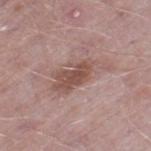<tbp_lesion>
<biopsy_status>not biopsied; imaged during a skin examination</biopsy_status>
<site>left thigh</site>
<image>
  <source>total-body photography crop</source>
  <field_of_view_mm>15</field_of_view_mm>
</image>
<lighting>white-light</lighting>
<automated_metrics>
  <cielab_L>52</cielab_L>
  <cielab_a>20</cielab_a>
  <cielab_b>23</cielab_b>
  <vs_skin_darker_L>10.0</vs_skin_darker_L>
  <vs_skin_contrast_norm>7.5</vs_skin_contrast_norm>
  <border_irregularity_0_10>6.5</border_irregularity_0_10>
  <color_variation_0_10>3.0</color_variation_0_10>
  <nevus_likeness_0_100>35</nevus_likeness_0_100>
  <lesion_detection_confidence_0_100>100</lesion_detection_confidence_0_100>
</automated_metrics>
<patient>
  <sex>male</sex>
  <age_approx>50</age_approx>
</patient>
<lesion_size>
  <long_diameter_mm_approx>6.5</long_diameter_mm_approx>
</lesion_size>
</tbp_lesion>